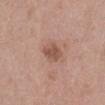Clinical impression: No biopsy was performed on this lesion — it was imaged during a full skin examination and was not determined to be concerning. Context: The total-body-photography lesion software estimated a border-irregularity index near 2/10 and a peripheral color-asymmetry measure near 1. The software also gave a classifier nevus-likeness of about 30/100. A region of skin cropped from a whole-body photographic capture, roughly 15 mm wide. A female patient aged around 70. This is a white-light tile. The lesion is located on the right lower leg. Longest diameter approximately 3 mm.